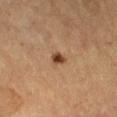  biopsy_status: not biopsied; imaged during a skin examination
  site: right thigh
  lighting: cross-polarized
  patient:
    sex: female
    age_approx: 70
  image:
    source: total-body photography crop
    field_of_view_mm: 15